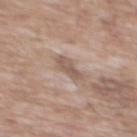Assessment:
This lesion was catalogued during total-body skin photography and was not selected for biopsy.